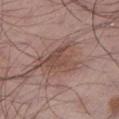Imaged during a routine full-body skin examination; the lesion was not biopsied and no histopathology is available. A roughly 15 mm field-of-view crop from a total-body skin photograph. A male patient aged approximately 55. Located on the left lower leg. The lesion-visualizer software estimated a border-irregularity rating of about 6/10. Measured at roughly 6 mm in maximum diameter. Imaged with white-light lighting.